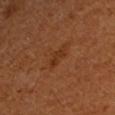Q: Was this lesion biopsied?
A: imaged on a skin check; not biopsied
Q: What lighting was used for the tile?
A: cross-polarized
Q: How was this image acquired?
A: total-body-photography crop, ~15 mm field of view
Q: Where on the body is the lesion?
A: the left upper arm
Q: Patient demographics?
A: female
Q: Automated lesion metrics?
A: an average lesion color of about L≈34 a*≈25 b*≈35 (CIELAB), about 6 CIELAB-L* units darker than the surrounding skin, and a normalized lesion–skin contrast near 6.5; a nevus-likeness score of about 0/100 and lesion-presence confidence of about 100/100
Q: Lesion size?
A: about 2.5 mm